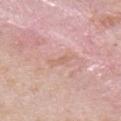{
  "biopsy_status": "not biopsied; imaged during a skin examination",
  "automated_metrics": {
    "eccentricity": 0.95,
    "shape_asymmetry": 0.25,
    "cielab_L": 64,
    "cielab_a": 21,
    "cielab_b": 28,
    "vs_skin_darker_L": 6.0,
    "vs_skin_contrast_norm": 5.0,
    "nevus_likeness_0_100": 0
  },
  "patient": {
    "sex": "male",
    "age_approx": 40
  },
  "site": "front of the torso",
  "lighting": "white-light",
  "image": {
    "source": "total-body photography crop",
    "field_of_view_mm": 15
  },
  "lesion_size": {
    "long_diameter_mm_approx": 2.5
  }
}